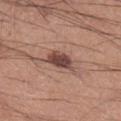Q: Was a biopsy performed?
A: catalogued during a skin exam; not biopsied
Q: Where on the body is the lesion?
A: the right lower leg
Q: What lighting was used for the tile?
A: white-light
Q: Patient demographics?
A: male, aged around 55
Q: What kind of image is this?
A: ~15 mm crop, total-body skin-cancer survey
Q: Lesion size?
A: about 3.5 mm